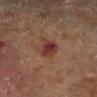The lesion is located on the right lower leg. A male subject, aged approximately 70. Cropped from a whole-body photographic skin survey; the tile spans about 15 mm.